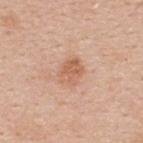The lesion was photographed on a routine skin check and not biopsied; there is no pathology result. A male subject, in their mid-30s. This is a white-light tile. Cropped from a total-body skin-imaging series; the visible field is about 15 mm. The recorded lesion diameter is about 3 mm. The lesion is located on the upper back. The total-body-photography lesion software estimated an average lesion color of about L≈61 a*≈23 b*≈33 (CIELAB) and a lesion–skin lightness drop of about 9. It also reported a border-irregularity index near 2/10, a within-lesion color-variation index near 3/10, and peripheral color asymmetry of about 1. It also reported a classifier nevus-likeness of about 10/100 and lesion-presence confidence of about 100/100.